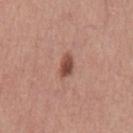Clinical impression: The lesion was tiled from a total-body skin photograph and was not biopsied. Image and clinical context: From the front of the torso. The tile uses white-light illumination. Cropped from a total-body skin-imaging series; the visible field is about 15 mm. The patient is a male aged 38 to 42.